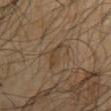This lesion was catalogued during total-body skin photography and was not selected for biopsy. Longest diameter approximately 4 mm. A close-up tile cropped from a whole-body skin photograph, about 15 mm across. The subject is a male in their mid-60s. Located on the mid back.